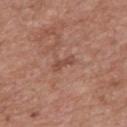Assessment: Part of a total-body skin-imaging series; this lesion was reviewed on a skin check and was not flagged for biopsy. Clinical summary: A lesion tile, about 15 mm wide, cut from a 3D total-body photograph. Located on the back. A male patient, roughly 50 years of age. Automated tile analysis of the lesion measured an eccentricity of roughly 0.95 and a symmetry-axis asymmetry near 0.45. The software also gave roughly 8 lightness units darker than nearby skin and a normalized border contrast of about 6. It also reported a border-irregularity index near 5/10, a within-lesion color-variation index near 0/10, and radial color variation of about 0. It also reported a nevus-likeness score of about 0/100 and lesion-presence confidence of about 100/100.